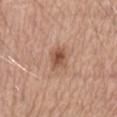Findings:
– follow-up · no biopsy performed (imaged during a skin exam)
– diameter · ~3 mm (longest diameter)
– imaging modality · ~15 mm tile from a whole-body skin photo
– patient · male, in their mid- to late 60s
– location · the front of the torso
– lighting · white-light